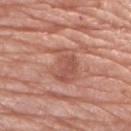* follow-up · total-body-photography surveillance lesion; no biopsy
* automated lesion analysis · an outline eccentricity of about 0.6 (0 = round, 1 = elongated) and a shape-asymmetry score of about 0.4 (0 = symmetric); a lesion color around L≈54 a*≈25 b*≈28 in CIELAB, about 9 CIELAB-L* units darker than the surrounding skin, and a normalized border contrast of about 6; a border-irregularity index near 5.5/10, a color-variation rating of about 3/10, and peripheral color asymmetry of about 1; a classifier nevus-likeness of about 0/100 and a detector confidence of about 95 out of 100 that the crop contains a lesion
* diameter · ~4 mm (longest diameter)
* body site · the right upper arm
* acquisition · 15 mm crop, total-body photography
* patient · female, in their mid- to late 70s